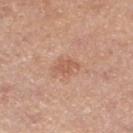Notes:
• biopsy status · total-body-photography surveillance lesion; no biopsy
• patient · female, in their 40s
• imaging modality · ~15 mm tile from a whole-body skin photo
• size · ≈2.5 mm
• location · the left lower leg
• tile lighting · white-light
• automated lesion analysis · a lesion color around L≈58 a*≈23 b*≈32 in CIELAB, a lesion–skin lightness drop of about 8, and a normalized lesion–skin contrast near 5.5; a nevus-likeness score of about 35/100 and a detector confidence of about 100 out of 100 that the crop contains a lesion